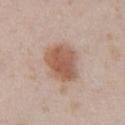<lesion>
  <biopsy_status>not biopsied; imaged during a skin examination</biopsy_status>
  <automated_metrics>
    <area_mm2_approx>15.0</area_mm2_approx>
    <border_irregularity_0_10>1.5</border_irregularity_0_10>
    <color_variation_0_10>4.0</color_variation_0_10>
    <peripheral_color_asymmetry>1.0</peripheral_color_asymmetry>
  </automated_metrics>
  <image>
    <source>total-body photography crop</source>
    <field_of_view_mm>15</field_of_view_mm>
  </image>
  <site>chest</site>
  <patient>
    <sex>female</sex>
    <age_approx>30</age_approx>
  </patient>
</lesion>